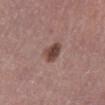- notes · total-body-photography surveillance lesion; no biopsy
- location · the left lower leg
- subject · male, about 45 years old
- image · ~15 mm crop, total-body skin-cancer survey